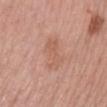Q: Was a biopsy performed?
A: imaged on a skin check; not biopsied
Q: Where on the body is the lesion?
A: the leg
Q: What kind of image is this?
A: ~15 mm tile from a whole-body skin photo
Q: Patient demographics?
A: female, approximately 65 years of age